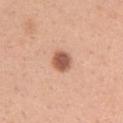{
  "site": "left upper arm",
  "patient": {
    "sex": "female",
    "age_approx": 30
  },
  "image": {
    "source": "total-body photography crop",
    "field_of_view_mm": 15
  },
  "lesion_size": {
    "long_diameter_mm_approx": 2.5
  },
  "automated_metrics": {
    "area_mm2_approx": 5.0,
    "shape_asymmetry": 0.2,
    "nevus_likeness_0_100": 100
  },
  "lighting": "white-light"
}